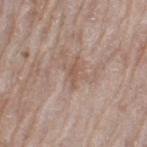Captured during whole-body skin photography for melanoma surveillance; the lesion was not biopsied. The patient is a female about 75 years old. The lesion is located on the left thigh. Cropped from a total-body skin-imaging series; the visible field is about 15 mm. An algorithmic analysis of the crop reported a mean CIELAB color near L≈54 a*≈17 b*≈26, roughly 7 lightness units darker than nearby skin, and a normalized lesion–skin contrast near 5.5. It also reported a border-irregularity rating of about 4/10 and peripheral color asymmetry of about 0. And it measured an automated nevus-likeness rating near 0 out of 100 and a detector confidence of about 90 out of 100 that the crop contains a lesion.